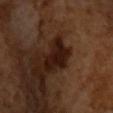No biopsy was performed on this lesion — it was imaged during a full skin examination and was not determined to be concerning.
Automated image analysis of the tile measured a lesion area of about 9.5 mm², an outline eccentricity of about 0.8 (0 = round, 1 = elongated), and a shape-asymmetry score of about 0.45 (0 = symmetric). The analysis additionally found an average lesion color of about L≈17 a*≈18 b*≈21 (CIELAB), roughly 11 lightness units darker than nearby skin, and a normalized border contrast of about 13.
Approximately 4.5 mm at its widest.
The subject is a male about 65 years old.
This is a cross-polarized tile.
A close-up tile cropped from a whole-body skin photograph, about 15 mm across.